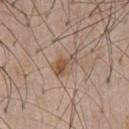Impression: The lesion was tiled from a total-body skin photograph and was not biopsied. Acquisition and patient details: The tile uses white-light illumination. A male subject aged 58–62. Located on the chest. Cropped from a whole-body photographic skin survey; the tile spans about 15 mm.